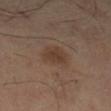Assessment:
No biopsy was performed on this lesion — it was imaged during a full skin examination and was not determined to be concerning.
Context:
A close-up tile cropped from a whole-body skin photograph, about 15 mm across. From the right leg. Measured at roughly 3 mm in maximum diameter. A female subject, aged 58–62. Imaged with cross-polarized lighting. The lesion-visualizer software estimated a lesion area of about 6 mm² and a shape-asymmetry score of about 0.25 (0 = symmetric). And it measured a lesion color around L≈38 a*≈17 b*≈26 in CIELAB, about 7 CIELAB-L* units darker than the surrounding skin, and a normalized border contrast of about 6.5. The analysis additionally found border irregularity of about 2.5 on a 0–10 scale and a within-lesion color-variation index near 2/10.